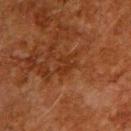No biopsy was performed on this lesion — it was imaged during a full skin examination and was not determined to be concerning. The patient is a male roughly 60 years of age. On the upper back. The tile uses cross-polarized illumination. A lesion tile, about 15 mm wide, cut from a 3D total-body photograph. Approximately 2.5 mm at its widest.